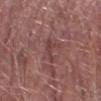| key | value |
|---|---|
| follow-up | imaged on a skin check; not biopsied |
| image source | ~15 mm tile from a whole-body skin photo |
| site | the right forearm |
| automated lesion analysis | a lesion area of about 4 mm², an eccentricity of roughly 0.9, and two-axis asymmetry of about 0.45; a mean CIELAB color near L≈41 a*≈23 b*≈21, roughly 7 lightness units darker than nearby skin, and a normalized lesion–skin contrast near 6; a border-irregularity index near 5.5/10, a color-variation rating of about 1/10, and a peripheral color-asymmetry measure near 0.5; a classifier nevus-likeness of about 0/100 and a lesion-detection confidence of about 70/100 |
| diameter | ≈3 mm |
| subject | male, about 80 years old |